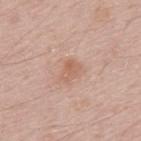  biopsy_status: not biopsied; imaged during a skin examination
  image:
    source: total-body photography crop
    field_of_view_mm: 15
  lighting: white-light
  patient:
    sex: male
    age_approx: 50
  site: mid back
  automated_metrics:
    area_mm2_approx: 3.5
    eccentricity: 0.75
    shape_asymmetry: 0.3
    vs_skin_darker_L: 7.0
    border_irregularity_0_10: 2.5
    color_variation_0_10: 2.0
    peripheral_color_asymmetry: 0.5
    lesion_detection_confidence_0_100: 100
  lesion_size:
    long_diameter_mm_approx: 2.5